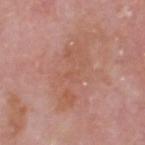{"site": "head or neck", "lighting": "white-light", "image": {"source": "total-body photography crop", "field_of_view_mm": 15}, "patient": {"sex": "male", "age_approx": 60}, "lesion_size": {"long_diameter_mm_approx": 7.0}}